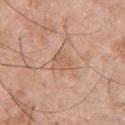workup = catalogued during a skin exam; not biopsied
automated metrics = border irregularity of about 3.5 on a 0–10 scale, a within-lesion color-variation index near 2.5/10, and radial color variation of about 1; lesion-presence confidence of about 95/100
acquisition = ~15 mm crop, total-body skin-cancer survey
location = the upper back
tile lighting = white-light
patient = male, approximately 55 years of age